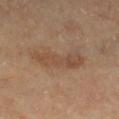biopsy status = imaged on a skin check; not biopsied | body site = the right lower leg | patient = female, aged 58–62 | image = ~15 mm tile from a whole-body skin photo.